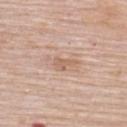{
  "biopsy_status": "not biopsied; imaged during a skin examination",
  "patient": {
    "sex": "female",
    "age_approx": 70
  },
  "image": {
    "source": "total-body photography crop",
    "field_of_view_mm": 15
  },
  "site": "upper back"
}